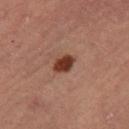No biopsy was performed on this lesion — it was imaged during a full skin examination and was not determined to be concerning. The lesion is located on the right thigh. A female patient, about 60 years old. About 3 mm across. A 15 mm close-up tile from a total-body photography series done for melanoma screening.